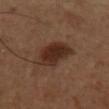biopsy status: no biopsy performed (imaged during a skin exam)
diameter: ≈5.5 mm
acquisition: 15 mm crop, total-body photography
site: the left upper arm
subject: male, aged 48 to 52
illumination: cross-polarized